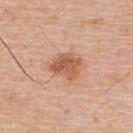notes = total-body-photography surveillance lesion; no biopsy.